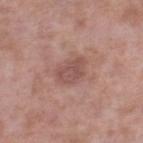follow-up = no biopsy performed (imaged during a skin exam) | size = about 3.5 mm | image source = ~15 mm crop, total-body skin-cancer survey | patient = male, aged around 55 | lighting = white-light | location = the right lower leg.